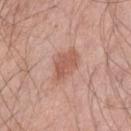| key | value |
|---|---|
| automated lesion analysis | a mean CIELAB color near L≈58 a*≈23 b*≈28, about 9 CIELAB-L* units darker than the surrounding skin, and a lesion-to-skin contrast of about 6.5 (normalized; higher = more distinct); internal color variation of about 2.5 on a 0–10 scale |
| subject | male, aged 43 to 47 |
| diameter | ~4.5 mm (longest diameter) |
| location | the left upper arm |
| illumination | white-light |
| acquisition | ~15 mm tile from a whole-body skin photo |